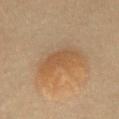biopsy_status: not biopsied; imaged during a skin examination
lighting: cross-polarized
image:
  source: total-body photography crop
  field_of_view_mm: 15
lesion_size:
  long_diameter_mm_approx: 5.0
site: chest
patient:
  sex: female
  age_approx: 60
automated_metrics:
  cielab_L: 48
  cielab_a: 17
  cielab_b: 34
  vs_skin_darker_L: 6.0
  vs_skin_contrast_norm: 5.0
  border_irregularity_0_10: 4.0